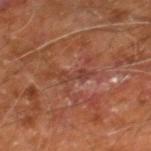notes — imaged on a skin check; not biopsied | lesion size — ~3.5 mm (longest diameter) | anatomic site — the right leg | patient — male, aged approximately 60 | acquisition — ~15 mm crop, total-body skin-cancer survey | lighting — cross-polarized.